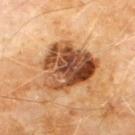Q: Was a biopsy performed?
A: catalogued during a skin exam; not biopsied
Q: What is the anatomic site?
A: the chest
Q: Patient demographics?
A: male, aged 58 to 62
Q: What kind of image is this?
A: ~15 mm crop, total-body skin-cancer survey